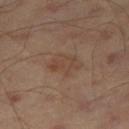Assessment: This lesion was catalogued during total-body skin photography and was not selected for biopsy. Image and clinical context: The lesion's longest dimension is about 4 mm. The subject is a male in their mid-40s. Cropped from a total-body skin-imaging series; the visible field is about 15 mm. The lesion is located on the right thigh.